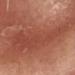Impression: Recorded during total-body skin imaging; not selected for excision or biopsy. Background: Measured at roughly 10.5 mm in maximum diameter. This is a white-light tile. A male patient, aged 68–72. A 15 mm crop from a total-body photograph taken for skin-cancer surveillance. The total-body-photography lesion software estimated an area of roughly 50 mm², an eccentricity of roughly 0.75, and a symmetry-axis asymmetry near 0.5. It also reported roughly 7 lightness units darker than nearby skin and a lesion-to-skin contrast of about 5.5 (normalized; higher = more distinct). It also reported a border-irregularity rating of about 7/10, internal color variation of about 3 on a 0–10 scale, and peripheral color asymmetry of about 1. The analysis additionally found an automated nevus-likeness rating near 0 out of 100 and a lesion-detection confidence of about 75/100. Located on the head or neck.